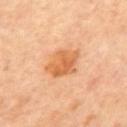biopsy status = catalogued during a skin exam; not biopsied | tile lighting = cross-polarized | anatomic site = the mid back | image source = ~15 mm tile from a whole-body skin photo | lesion size = about 4.5 mm | subject = male, aged 58–62.